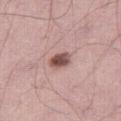Clinical impression:
This lesion was catalogued during total-body skin photography and was not selected for biopsy.
Image and clinical context:
From the leg. This image is a 15 mm lesion crop taken from a total-body photograph. The patient is a male approximately 45 years of age.